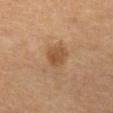Captured during whole-body skin photography for melanoma surveillance; the lesion was not biopsied. A roughly 15 mm field-of-view crop from a total-body skin photograph. The subject is a female about 60 years old. Located on the abdomen.